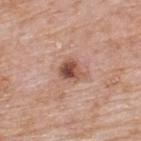No biopsy was performed on this lesion — it was imaged during a full skin examination and was not determined to be concerning. Approximately 3 mm at its widest. On the upper back. Cropped from a whole-body photographic skin survey; the tile spans about 15 mm. The tile uses white-light illumination. A male subject, aged 73 to 77. The total-body-photography lesion software estimated an area of roughly 5 mm², an outline eccentricity of about 0.55 (0 = round, 1 = elongated), and two-axis asymmetry of about 0.3. The software also gave a border-irregularity index near 2.5/10 and peripheral color asymmetry of about 3.